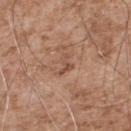| field | value |
|---|---|
| follow-up | imaged on a skin check; not biopsied |
| illumination | white-light illumination |
| patient | male, aged around 55 |
| lesion size | about 2.5 mm |
| image source | 15 mm crop, total-body photography |
| anatomic site | the upper back |
| image-analysis metrics | a lesion area of about 2.5 mm², an outline eccentricity of about 0.85 (0 = round, 1 = elongated), and a shape-asymmetry score of about 0.6 (0 = symmetric); a border-irregularity rating of about 6/10, a within-lesion color-variation index near 0/10, and a peripheral color-asymmetry measure near 0; an automated nevus-likeness rating near 0 out of 100 and a detector confidence of about 100 out of 100 that the crop contains a lesion |